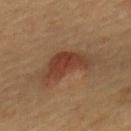Case summary:
- workup · imaged on a skin check; not biopsied
- location · the mid back
- size · about 5 mm
- acquisition · ~15 mm crop, total-body skin-cancer survey
- illumination · cross-polarized illumination
- subject · female, aged 58–62